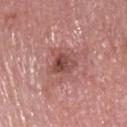<case>
<lesion_size>
  <long_diameter_mm_approx>3.5</long_diameter_mm_approx>
</lesion_size>
<patient>
  <sex>male</sex>
  <age_approx>85+</age_approx>
</patient>
<site>head or neck</site>
<image>
  <source>total-body photography crop</source>
  <field_of_view_mm>15</field_of_view_mm>
</image>
<lighting>white-light</lighting>
</case>